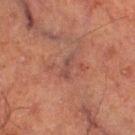{
  "biopsy_status": "not biopsied; imaged during a skin examination",
  "lighting": "cross-polarized",
  "site": "left thigh",
  "patient": {
    "sex": "male",
    "age_approx": 65
  },
  "image": {
    "source": "total-body photography crop",
    "field_of_view_mm": 15
  }
}